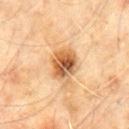Assessment: This lesion was catalogued during total-body skin photography and was not selected for biopsy. Background: The subject is a male aged around 60. Measured at roughly 4.5 mm in maximum diameter. Located on the chest. Automated tile analysis of the lesion measured a footprint of about 11 mm² and a shape-asymmetry score of about 0.2 (0 = symmetric). The software also gave a nevus-likeness score of about 95/100 and a detector confidence of about 100 out of 100 that the crop contains a lesion. Cropped from a total-body skin-imaging series; the visible field is about 15 mm.